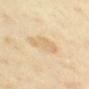Captured during whole-body skin photography for melanoma surveillance; the lesion was not biopsied.
The patient is a female in their mid- to late 30s.
A close-up tile cropped from a whole-body skin photograph, about 15 mm across.
From the upper back.